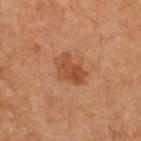Acquisition and patient details:
On the upper back. The recorded lesion diameter is about 4 mm. A close-up tile cropped from a whole-body skin photograph, about 15 mm across. Imaged with cross-polarized lighting. A female subject, aged approximately 60.